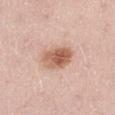notes: imaged on a skin check; not biopsied | subject: male, aged 48 to 52 | lighting: white-light | acquisition: ~15 mm tile from a whole-body skin photo | site: the right thigh | automated metrics: an area of roughly 10 mm², an outline eccentricity of about 0.75 (0 = round, 1 = elongated), and a shape-asymmetry score of about 0.2 (0 = symmetric); a classifier nevus-likeness of about 95/100 and a detector confidence of about 100 out of 100 that the crop contains a lesion | size: ~4 mm (longest diameter).